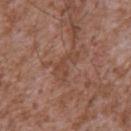follow-up: imaged on a skin check; not biopsied | site: the chest | acquisition: ~15 mm tile from a whole-body skin photo | subject: male, aged around 45.